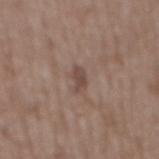The lesion was photographed on a routine skin check and not biopsied; there is no pathology result.
The lesion is on the back.
Longest diameter approximately 3 mm.
A male patient, about 50 years old.
Imaged with white-light lighting.
A close-up tile cropped from a whole-body skin photograph, about 15 mm across.